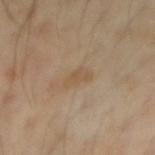Findings:
* workup · catalogued during a skin exam; not biopsied
* illumination · cross-polarized
* image · ~15 mm crop, total-body skin-cancer survey
* anatomic site · the right forearm
* TBP lesion metrics · a lesion area of about 3.5 mm², an outline eccentricity of about 0.85 (0 = round, 1 = elongated), and a symmetry-axis asymmetry near 0.35; a border-irregularity index near 3.5/10 and internal color variation of about 2 on a 0–10 scale
* patient · male, aged 43 to 47